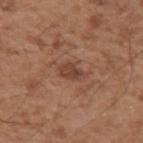Assessment:
Part of a total-body skin-imaging series; this lesion was reviewed on a skin check and was not flagged for biopsy.